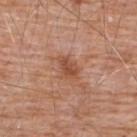Imaged with white-light lighting. A male patient, about 80 years old. Automated tile analysis of the lesion measured a lesion color around L≈50 a*≈24 b*≈32 in CIELAB and a lesion–skin lightness drop of about 9. And it measured border irregularity of about 3 on a 0–10 scale, a within-lesion color-variation index near 2.5/10, and peripheral color asymmetry of about 1. The software also gave an automated nevus-likeness rating near 0 out of 100 and a lesion-detection confidence of about 100/100. Located on the upper back. This image is a 15 mm lesion crop taken from a total-body photograph.